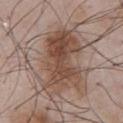workup: total-body-photography surveillance lesion; no biopsy | patient: male, roughly 55 years of age | body site: the chest | imaging modality: total-body-photography crop, ~15 mm field of view | lighting: white-light | diameter: about 8.5 mm.